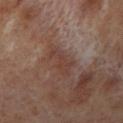Assessment: Part of a total-body skin-imaging series; this lesion was reviewed on a skin check and was not flagged for biopsy. Image and clinical context: The lesion is on the left lower leg. A 15 mm crop from a total-body photograph taken for skin-cancer surveillance. The total-body-photography lesion software estimated roughly 7 lightness units darker than nearby skin and a normalized lesion–skin contrast near 6. Measured at roughly 4.5 mm in maximum diameter. The subject is a female about 50 years old. Imaged with cross-polarized lighting.